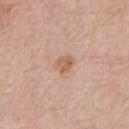This lesion was catalogued during total-body skin photography and was not selected for biopsy.
A male patient aged approximately 75.
The tile uses white-light illumination.
A 15 mm close-up extracted from a 3D total-body photography capture.
From the chest.